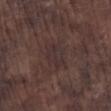illumination=white-light
subject=male, about 75 years old
anatomic site=the left lower leg
image=~15 mm tile from a whole-body skin photo